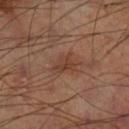Part of a total-body skin-imaging series; this lesion was reviewed on a skin check and was not flagged for biopsy. A close-up tile cropped from a whole-body skin photograph, about 15 mm across. The lesion's longest dimension is about 3 mm. Imaged with cross-polarized lighting. From the right thigh.